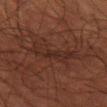follow-up: no biopsy performed (imaged during a skin exam) | imaging modality: ~15 mm tile from a whole-body skin photo | anatomic site: the lower back | subject: male, aged around 55 | lesion diameter: ≈6 mm.